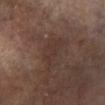workup = imaged on a skin check; not biopsied
location = the right lower leg
imaging modality = ~15 mm crop, total-body skin-cancer survey
patient = female, roughly 75 years of age
tile lighting = cross-polarized
lesion size = ~5 mm (longest diameter)